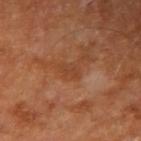notes: catalogued during a skin exam; not biopsied | location: the right upper arm | image: 15 mm crop, total-body photography | size: ≈2.5 mm | subject: male, in their 70s.